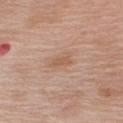Findings:
* biopsy status: total-body-photography surveillance lesion; no biopsy
* subject: male, about 65 years old
* TBP lesion metrics: a lesion area of about 3 mm²; a lesion–skin lightness drop of about 7; internal color variation of about 0 on a 0–10 scale and a peripheral color-asymmetry measure near 0
* imaging modality: total-body-photography crop, ~15 mm field of view
* location: the right upper arm
* tile lighting: white-light illumination